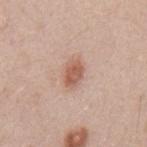biopsy status: catalogued during a skin exam; not biopsied
subject: male, in their 50s
site: the back
image source: ~15 mm crop, total-body skin-cancer survey